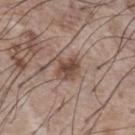No biopsy was performed on this lesion — it was imaged during a full skin examination and was not determined to be concerning. A lesion tile, about 15 mm wide, cut from a 3D total-body photograph. From the chest. Approximately 3.5 mm at its widest. The tile uses white-light illumination. A male patient, aged approximately 75.